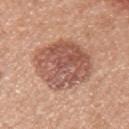subject: female, about 50 years old | image: total-body-photography crop, ~15 mm field of view | body site: the left upper arm.